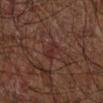Part of a total-body skin-imaging series; this lesion was reviewed on a skin check and was not flagged for biopsy.
This is a cross-polarized tile.
A lesion tile, about 15 mm wide, cut from a 3D total-body photograph.
On the right forearm.
A male subject, about 70 years old.
Automated tile analysis of the lesion measured a footprint of about 3 mm², a shape eccentricity near 0.9, and two-axis asymmetry of about 0.35.
The recorded lesion diameter is about 2.5 mm.